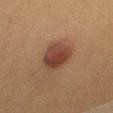Recorded during total-body skin imaging; not selected for excision or biopsy. The recorded lesion diameter is about 4.5 mm. Located on the right thigh. This image is a 15 mm lesion crop taken from a total-body photograph. This is a cross-polarized tile. The subject is a female aged approximately 50.